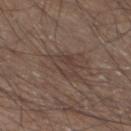biopsy status: catalogued during a skin exam; not biopsied